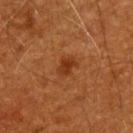The lesion was photographed on a routine skin check and not biopsied; there is no pathology result. A 15 mm close-up extracted from a 3D total-body photography capture. A male subject, in their 60s. The lesion-visualizer software estimated a lesion area of about 4 mm², an outline eccentricity of about 0.7 (0 = round, 1 = elongated), and a symmetry-axis asymmetry near 0.2. The lesion is located on the upper back. Captured under cross-polarized illumination.